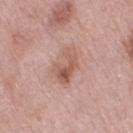Assessment: Part of a total-body skin-imaging series; this lesion was reviewed on a skin check and was not flagged for biopsy. Clinical summary: An algorithmic analysis of the crop reported a footprint of about 8.5 mm² and an eccentricity of roughly 0.8. The software also gave a mean CIELAB color near L≈57 a*≈21 b*≈26 and about 10 CIELAB-L* units darker than the surrounding skin. It also reported internal color variation of about 7 on a 0–10 scale and peripheral color asymmetry of about 3. A female patient aged 68–72. The lesion is on the right thigh. Approximately 4.5 mm at its widest. Imaged with white-light lighting. Cropped from a total-body skin-imaging series; the visible field is about 15 mm.